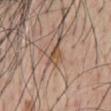workup: imaged on a skin check; not biopsied | image-analysis metrics: a lesion area of about 3.5 mm², a shape eccentricity near 0.8, and two-axis asymmetry of about 0.3; a border-irregularity index near 2.5/10, a within-lesion color-variation index near 4.5/10, and peripheral color asymmetry of about 1.5; a nevus-likeness score of about 0/100 | site: the front of the torso | tile lighting: white-light | subject: male, aged around 55 | imaging modality: total-body-photography crop, ~15 mm field of view.